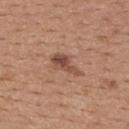Assessment:
Imaged during a routine full-body skin examination; the lesion was not biopsied and no histopathology is available.
Image and clinical context:
The lesion is on the upper back. Captured under white-light illumination. The subject is a female approximately 35 years of age. The recorded lesion diameter is about 4 mm. A lesion tile, about 15 mm wide, cut from a 3D total-body photograph.